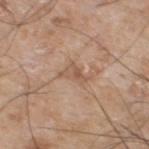Impression: This lesion was catalogued during total-body skin photography and was not selected for biopsy. Context: Cropped from a total-body skin-imaging series; the visible field is about 15 mm. Longest diameter approximately 3.5 mm. The lesion is on the left lower leg. The subject is a male aged approximately 60. The tile uses white-light illumination.